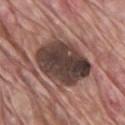Impression: Captured during whole-body skin photography for melanoma surveillance; the lesion was not biopsied. Image and clinical context: From the chest. A lesion tile, about 15 mm wide, cut from a 3D total-body photograph. The subject is a male in their mid-60s.